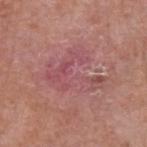biopsy_status: not biopsied; imaged during a skin examination
image:
  source: total-body photography crop
  field_of_view_mm: 15
site: arm
patient:
  sex: male
  age_approx: 55
automated_metrics:
  area_mm2_approx: 20.0
  eccentricity: 0.6
  nevus_likeness_0_100: 0
  lesion_detection_confidence_0_100: 95
lighting: white-light